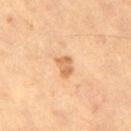follow-up: imaged on a skin check; not biopsied
lighting: cross-polarized
automated metrics: a lesion area of about 4.5 mm² and an eccentricity of roughly 0.75; border irregularity of about 3 on a 0–10 scale, internal color variation of about 2.5 on a 0–10 scale, and radial color variation of about 0.5; a classifier nevus-likeness of about 25/100 and a detector confidence of about 100 out of 100 that the crop contains a lesion
diameter: ≈2.5 mm
patient: male, aged 63 to 67
site: the left thigh
acquisition: total-body-photography crop, ~15 mm field of view